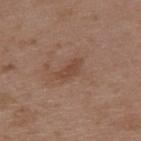notes=total-body-photography surveillance lesion; no biopsy | subject=male, approximately 50 years of age | location=the upper back | imaging modality=~15 mm crop, total-body skin-cancer survey.